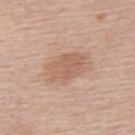Captured during whole-body skin photography for melanoma surveillance; the lesion was not biopsied.